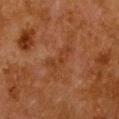<lesion>
<biopsy_status>not biopsied; imaged during a skin examination</biopsy_status>
<lighting>cross-polarized</lighting>
<lesion_size>
  <long_diameter_mm_approx>4.0</long_diameter_mm_approx>
</lesion_size>
<site>chest</site>
<patient>
  <sex>female</sex>
  <age_approx>50</age_approx>
</patient>
<image>
  <source>total-body photography crop</source>
  <field_of_view_mm>15</field_of_view_mm>
</image>
</lesion>